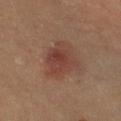Assessment:
The lesion was tiled from a total-body skin photograph and was not biopsied.
Image and clinical context:
An algorithmic analysis of the crop reported a border-irregularity rating of about 3/10, internal color variation of about 4 on a 0–10 scale, and a peripheral color-asymmetry measure near 1. The subject is aged 58–62. The lesion is located on the right lower leg. A 15 mm crop from a total-body photograph taken for skin-cancer surveillance.